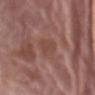biopsy status: no biopsy performed (imaged during a skin exam) | lesion size: ~2.5 mm (longest diameter) | illumination: white-light illumination | image source: ~15 mm crop, total-body skin-cancer survey | automated lesion analysis: a lesion–skin lightness drop of about 5 and a normalized lesion–skin contrast near 4.5; border irregularity of about 2 on a 0–10 scale, a color-variation rating of about 2.5/10, and peripheral color asymmetry of about 1 | subject: female, aged around 75 | body site: the front of the torso.